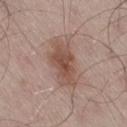| feature | finding |
|---|---|
| workup | imaged on a skin check; not biopsied |
| acquisition | ~15 mm crop, total-body skin-cancer survey |
| illumination | white-light |
| patient | male, approximately 55 years of age |
| lesion size | about 6 mm |
| anatomic site | the right thigh |